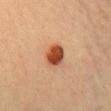Clinical summary:
Approximately 3 mm at its widest. A close-up tile cropped from a whole-body skin photograph, about 15 mm across. The lesion is located on the chest. The patient is a female aged approximately 45. Captured under cross-polarized illumination.